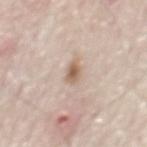| feature | finding |
|---|---|
| follow-up | imaged on a skin check; not biopsied |
| illumination | white-light |
| lesion diameter | ≈2.5 mm |
| automated metrics | two-axis asymmetry of about 0.25; a lesion color around L≈61 a*≈16 b*≈28 in CIELAB and a normalized lesion–skin contrast near 8; an automated nevus-likeness rating near 95 out of 100 and a lesion-detection confidence of about 100/100 |
| imaging modality | ~15 mm tile from a whole-body skin photo |
| patient | male, aged 78 to 82 |
| anatomic site | the mid back |